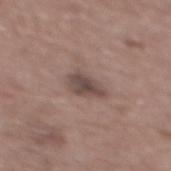No biopsy was performed on this lesion — it was imaged during a full skin examination and was not determined to be concerning. The lesion's longest dimension is about 4 mm. Imaged with white-light lighting. This image is a 15 mm lesion crop taken from a total-body photograph. Located on the mid back. The lesion-visualizer software estimated a footprint of about 6.5 mm² and a shape eccentricity near 0.8. The analysis additionally found a border-irregularity rating of about 2.5/10, a within-lesion color-variation index near 3/10, and peripheral color asymmetry of about 1. The analysis additionally found a nevus-likeness score of about 5/100 and a detector confidence of about 100 out of 100 that the crop contains a lesion. A male patient about 60 years old.